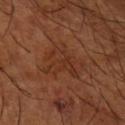The lesion was tiled from a total-body skin photograph and was not biopsied. Cropped from a whole-body photographic skin survey; the tile spans about 15 mm. A male patient in their mid- to late 60s. Approximately 5 mm at its widest. On the right forearm.